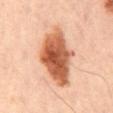Findings:
– anatomic site — the back
– imaging modality — ~15 mm crop, total-body skin-cancer survey
– subject — male, approximately 50 years of age